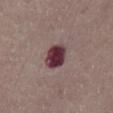The lesion was tiled from a total-body skin photograph and was not biopsied. This image is a 15 mm lesion crop taken from a total-body photograph. The lesion is on the abdomen. Longest diameter approximately 3.5 mm. A male patient, in their mid- to late 70s. Imaged with white-light lighting. An algorithmic analysis of the crop reported an area of roughly 8 mm² and a shape eccentricity near 0.55. And it measured an automated nevus-likeness rating near 0 out of 100 and a lesion-detection confidence of about 100/100.